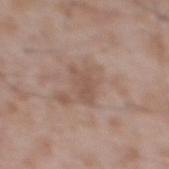<record>
<biopsy_status>not biopsied; imaged during a skin examination</biopsy_status>
<lighting>white-light</lighting>
<site>mid back</site>
<image>
  <source>total-body photography crop</source>
  <field_of_view_mm>15</field_of_view_mm>
</image>
<patient>
  <sex>male</sex>
  <age_approx>50</age_approx>
</patient>
<automated_metrics>
  <area_mm2_approx>6.0</area_mm2_approx>
  <eccentricity>0.8</eccentricity>
  <cielab_L>53</cielab_L>
  <cielab_a>17</cielab_a>
  <cielab_b>25</cielab_b>
  <vs_skin_darker_L>7.0</vs_skin_darker_L>
  <border_irregularity_0_10>3.0</border_irregularity_0_10>
  <color_variation_0_10>1.5</color_variation_0_10>
  <peripheral_color_asymmetry>0.5</peripheral_color_asymmetry>
  <nevus_likeness_0_100>0</nevus_likeness_0_100>
</automated_metrics>
<lesion_size>
  <long_diameter_mm_approx>3.5</long_diameter_mm_approx>
</lesion_size>
</record>